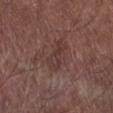The lesion was tiled from a total-body skin photograph and was not biopsied. Imaged with white-light lighting. The lesion is located on the right lower leg. A male patient aged approximately 55. Longest diameter approximately 3.5 mm. A close-up tile cropped from a whole-body skin photograph, about 15 mm across.